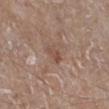biopsy_status: not biopsied; imaged during a skin examination
lesion_size:
  long_diameter_mm_approx: 2.5
image:
  source: total-body photography crop
  field_of_view_mm: 15
patient:
  sex: male
  age_approx: 80
lighting: white-light
automated_metrics:
  area_mm2_approx: 3.0
  shape_asymmetry: 0.35
  cielab_L: 49
  cielab_a: 18
  cielab_b: 26
  vs_skin_darker_L: 7.0
  vs_skin_contrast_norm: 6.0
site: right lower leg